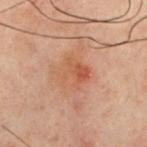notes — imaged on a skin check; not biopsied | lesion diameter — ≈3.5 mm | illumination — cross-polarized illumination | automated metrics — a lesion color around L≈42 a*≈19 b*≈27 in CIELAB and a lesion-to-skin contrast of about 5.5 (normalized; higher = more distinct); border irregularity of about 2 on a 0–10 scale and internal color variation of about 7 on a 0–10 scale | location — the chest | image — 15 mm crop, total-body photography | subject — male, aged 58 to 62.